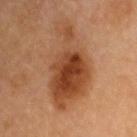{"image": {"source": "total-body photography crop", "field_of_view_mm": 15}, "patient": {"sex": "female", "age_approx": 55}, "site": "right upper arm", "lesion_size": {"long_diameter_mm_approx": 9.0}}